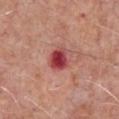Assessment:
Part of a total-body skin-imaging series; this lesion was reviewed on a skin check and was not flagged for biopsy.